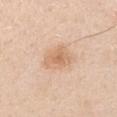Impression:
The lesion was tiled from a total-body skin photograph and was not biopsied.
Acquisition and patient details:
The subject is a male aged around 30. Approximately 4 mm at its widest. Captured under white-light illumination. A 15 mm close-up tile from a total-body photography series done for melanoma screening. On the left upper arm. The lesion-visualizer software estimated a mean CIELAB color near L≈68 a*≈19 b*≈34, roughly 9 lightness units darker than nearby skin, and a normalized border contrast of about 6. The analysis additionally found an automated nevus-likeness rating near 65 out of 100 and a detector confidence of about 100 out of 100 that the crop contains a lesion.